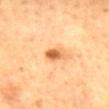notes: imaged on a skin check; not biopsied | image-analysis metrics: a footprint of about 4 mm² and two-axis asymmetry of about 0.2; an average lesion color of about L≈58 a*≈25 b*≈41 (CIELAB), about 14 CIELAB-L* units darker than the surrounding skin, and a normalized border contrast of about 9 | anatomic site: the mid back | tile lighting: cross-polarized illumination | patient: female, approximately 60 years of age | imaging modality: total-body-photography crop, ~15 mm field of view | lesion diameter: ~2.5 mm (longest diameter).